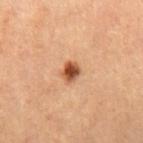Clinical impression: Captured during whole-body skin photography for melanoma surveillance; the lesion was not biopsied. Background: The lesion is located on the right thigh. Approximately 2.5 mm at its widest. This is a cross-polarized tile. A female subject, about 70 years old. A 15 mm crop from a total-body photograph taken for skin-cancer surveillance.